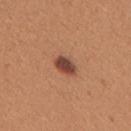| feature | finding |
|---|---|
| notes | catalogued during a skin exam; not biopsied |
| subject | female, roughly 30 years of age |
| imaging modality | 15 mm crop, total-body photography |
| location | the upper back |